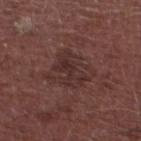Notes:
* tile lighting · white-light illumination
* image · ~15 mm crop, total-body skin-cancer survey
* image-analysis metrics · a detector confidence of about 100 out of 100 that the crop contains a lesion
* site · the left lower leg
* patient · male, roughly 75 years of age
* size · about 5 mm